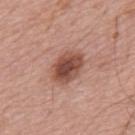notes: total-body-photography surveillance lesion; no biopsy.